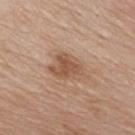Findings:
* notes: imaged on a skin check; not biopsied
* imaging modality: total-body-photography crop, ~15 mm field of view
* body site: the front of the torso
* subject: male, aged 78 to 82
* image-analysis metrics: an area of roughly 10 mm² and an eccentricity of roughly 0.7; a mean CIELAB color near L≈54 a*≈19 b*≈31, a lesion–skin lightness drop of about 10, and a lesion-to-skin contrast of about 7 (normalized; higher = more distinct); a border-irregularity rating of about 2.5/10, a color-variation rating of about 3.5/10, and radial color variation of about 1; a detector confidence of about 100 out of 100 that the crop contains a lesion